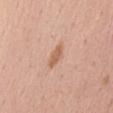workup — total-body-photography surveillance lesion; no biopsy
illumination — white-light
imaging modality — ~15 mm crop, total-body skin-cancer survey
anatomic site — the abdomen
patient — male, roughly 55 years of age